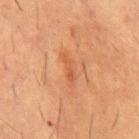No biopsy was performed on this lesion — it was imaged during a full skin examination and was not determined to be concerning. Captured under cross-polarized illumination. Cropped from a whole-body photographic skin survey; the tile spans about 15 mm. A male subject aged 53 to 57. The recorded lesion diameter is about 3.5 mm. The lesion is on the mid back.